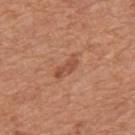The lesion was tiled from a total-body skin photograph and was not biopsied.
A 15 mm crop from a total-body photograph taken for skin-cancer surveillance.
A male patient, aged 63–67.
An algorithmic analysis of the crop reported a lesion area of about 3.5 mm², an outline eccentricity of about 0.95 (0 = round, 1 = elongated), and two-axis asymmetry of about 0.35. The software also gave a lesion–skin lightness drop of about 9 and a lesion-to-skin contrast of about 6.5 (normalized; higher = more distinct). The software also gave a border-irregularity index near 5/10, a within-lesion color-variation index near 0/10, and a peripheral color-asymmetry measure near 0. The software also gave a nevus-likeness score of about 20/100 and a lesion-detection confidence of about 100/100.
The lesion is located on the mid back.
Measured at roughly 3.5 mm in maximum diameter.
This is a white-light tile.